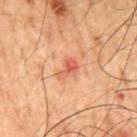Imaged during a routine full-body skin examination; the lesion was not biopsied and no histopathology is available. This is a cross-polarized tile. A male subject, roughly 50 years of age. On the back. A roughly 15 mm field-of-view crop from a total-body skin photograph. About 3 mm across.